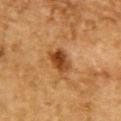Acquisition and patient details:
Imaged with cross-polarized lighting. On the upper back. Automated tile analysis of the lesion measured a shape eccentricity near 0.75. It also reported a lesion color around L≈38 a*≈22 b*≈36 in CIELAB and roughly 12 lightness units darker than nearby skin. The software also gave internal color variation of about 5 on a 0–10 scale and peripheral color asymmetry of about 1.5. A close-up tile cropped from a whole-body skin photograph, about 15 mm across. The patient is a male about 85 years old or older. Longest diameter approximately 3.5 mm.